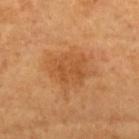Assessment:
Recorded during total-body skin imaging; not selected for excision or biopsy.
Context:
The lesion is on the left upper arm. A 15 mm close-up tile from a total-body photography series done for melanoma screening. Captured under cross-polarized illumination. A female subject, aged 63 to 67. The total-body-photography lesion software estimated a mean CIELAB color near L≈50 a*≈25 b*≈40, a lesion–skin lightness drop of about 7, and a lesion-to-skin contrast of about 5 (normalized; higher = more distinct). And it measured internal color variation of about 2.5 on a 0–10 scale. The analysis additionally found a nevus-likeness score of about 10/100.